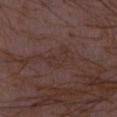biopsy status: no biopsy performed (imaged during a skin exam) | location: the abdomen | diameter: ≈3.5 mm | imaging modality: ~15 mm tile from a whole-body skin photo | automated lesion analysis: a lesion color around L≈32 a*≈16 b*≈20 in CIELAB and a lesion-to-skin contrast of about 4.5 (normalized; higher = more distinct); a border-irregularity rating of about 5.5/10 and a within-lesion color-variation index near 1.5/10 | patient: female, aged 28 to 32.